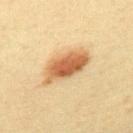biopsy status: imaged on a skin check; not biopsied | image-analysis metrics: a mean CIELAB color near L≈53 a*≈19 b*≈35 and a normalized border contrast of about 9.5; a classifier nevus-likeness of about 100/100 and a lesion-detection confidence of about 100/100 | imaging modality: ~15 mm tile from a whole-body skin photo | subject: female, aged approximately 40 | location: the back | size: ≈6 mm | lighting: cross-polarized.